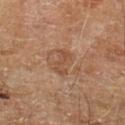Part of a total-body skin-imaging series; this lesion was reviewed on a skin check and was not flagged for biopsy. Measured at roughly 3.5 mm in maximum diameter. Imaged with cross-polarized lighting. The subject is a male aged approximately 70. From the left lower leg. A region of skin cropped from a whole-body photographic capture, roughly 15 mm wide.